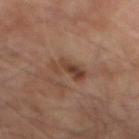Notes:
• notes: no biopsy performed (imaged during a skin exam)
• lesion size: ≈4 mm
• body site: the mid back
• tile lighting: cross-polarized illumination
• image: 15 mm crop, total-body photography
• subject: male, roughly 70 years of age